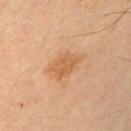Captured during whole-body skin photography for melanoma surveillance; the lesion was not biopsied.
Automated tile analysis of the lesion measured a mean CIELAB color near L≈48 a*≈19 b*≈33, about 7 CIELAB-L* units darker than the surrounding skin, and a normalized border contrast of about 6.5.
A 15 mm crop from a total-body photograph taken for skin-cancer surveillance.
On the left upper arm.
A male patient in their mid- to late 60s.
The recorded lesion diameter is about 3.5 mm.